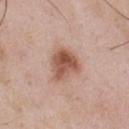Q: Was this lesion biopsied?
A: imaged on a skin check; not biopsied
Q: How was this image acquired?
A: ~15 mm tile from a whole-body skin photo
Q: Patient demographics?
A: male, aged 53–57
Q: How was the tile lit?
A: white-light illumination
Q: Where on the body is the lesion?
A: the chest